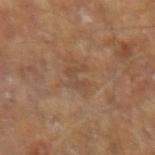follow-up: no biopsy performed (imaged during a skin exam) | diameter: ~2.5 mm (longest diameter) | TBP lesion metrics: a lesion color around L≈45 a*≈18 b*≈28 in CIELAB, about 6 CIELAB-L* units darker than the surrounding skin, and a lesion-to-skin contrast of about 5 (normalized; higher = more distinct) | body site: the arm | imaging modality: ~15 mm crop, total-body skin-cancer survey | tile lighting: cross-polarized | subject: male, roughly 65 years of age.